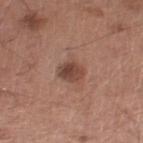Assessment: Part of a total-body skin-imaging series; this lesion was reviewed on a skin check and was not flagged for biopsy. Clinical summary: A male subject about 65 years old. Located on the leg. This image is a 15 mm lesion crop taken from a total-body photograph. Imaged with white-light lighting. About 2.5 mm across.